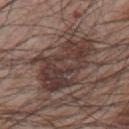Assessment:
Captured during whole-body skin photography for melanoma surveillance; the lesion was not biopsied.
Background:
Cropped from a total-body skin-imaging series; the visible field is about 15 mm. The lesion is located on the upper back. The lesion's longest dimension is about 7 mm. A male patient, in their mid- to late 60s. An algorithmic analysis of the crop reported an area of roughly 24 mm², an outline eccentricity of about 0.8 (0 = round, 1 = elongated), and two-axis asymmetry of about 0.35. The analysis additionally found an average lesion color of about L≈36 a*≈16 b*≈20 (CIELAB), a lesion–skin lightness drop of about 10, and a normalized lesion–skin contrast near 9. And it measured a lesion-detection confidence of about 65/100. This is a white-light tile.